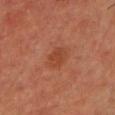Clinical summary: From the upper back. Approximately 3 mm at its widest. An algorithmic analysis of the crop reported a lesion color around L≈39 a*≈25 b*≈31 in CIELAB, roughly 6 lightness units darker than nearby skin, and a normalized border contrast of about 5.5. The software also gave a border-irregularity index near 1.5/10 and internal color variation of about 1.5 on a 0–10 scale. A male subject, aged 58 to 62. A 15 mm crop from a total-body photograph taken for skin-cancer surveillance. Imaged with cross-polarized lighting.